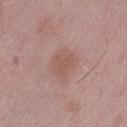No biopsy was performed on this lesion — it was imaged during a full skin examination and was not determined to be concerning. This is a white-light tile. A 15 mm close-up tile from a total-body photography series done for melanoma screening. Located on the leg. A male subject, aged around 50.